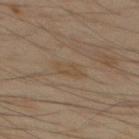Imaged during a routine full-body skin examination; the lesion was not biopsied and no histopathology is available. A male patient aged around 50. The lesion is located on the back. Longest diameter approximately 3 mm. A close-up tile cropped from a whole-body skin photograph, about 15 mm across.